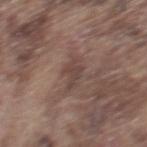Q: Was a biopsy performed?
A: catalogued during a skin exam; not biopsied
Q: How was this image acquired?
A: ~15 mm tile from a whole-body skin photo
Q: What are the patient's age and sex?
A: male, roughly 75 years of age
Q: Lesion location?
A: the upper back